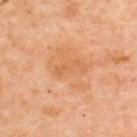image=15 mm crop, total-body photography
lesion diameter=~3.5 mm (longest diameter)
subject=male, aged approximately 60
anatomic site=the upper back
lighting=cross-polarized illumination
automated lesion analysis=an eccentricity of roughly 0.9 and two-axis asymmetry of about 0.45; a within-lesion color-variation index near 0/10 and peripheral color asymmetry of about 0; a nevus-likeness score of about 0/100 and a detector confidence of about 100 out of 100 that the crop contains a lesion